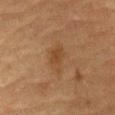Clinical summary:
This is a cross-polarized tile. A 15 mm close-up tile from a total-body photography series done for melanoma screening. An algorithmic analysis of the crop reported an area of roughly 6 mm² and two-axis asymmetry of about 0.3. And it measured a lesion color around L≈39 a*≈17 b*≈31 in CIELAB and roughly 6 lightness units darker than nearby skin. The analysis additionally found a border-irregularity index near 3/10, a within-lesion color-variation index near 2.5/10, and radial color variation of about 0.5. The software also gave a nevus-likeness score of about 5/100 and lesion-presence confidence of about 100/100. The lesion is located on the front of the torso. The subject is a male aged around 85 or older. About 3.5 mm across.